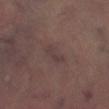biopsy status: catalogued during a skin exam; not biopsied | location: the left lower leg | TBP lesion metrics: a lesion color around L≈35 a*≈14 b*≈15 in CIELAB, about 5 CIELAB-L* units darker than the surrounding skin, and a lesion-to-skin contrast of about 6 (normalized; higher = more distinct); border irregularity of about 4 on a 0–10 scale, a within-lesion color-variation index near 0/10, and peripheral color asymmetry of about 0; a nevus-likeness score of about 0/100 | lighting: cross-polarized illumination | imaging modality: ~15 mm tile from a whole-body skin photo | subject: male, aged around 65.